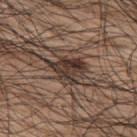Clinical impression:
Part of a total-body skin-imaging series; this lesion was reviewed on a skin check and was not flagged for biopsy.
Acquisition and patient details:
Captured under white-light illumination. The lesion's longest dimension is about 4 mm. From the upper back. A lesion tile, about 15 mm wide, cut from a 3D total-body photograph. A male patient aged 68–72.